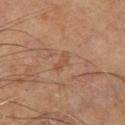The lesion is on the right leg.
A male subject, aged 58 to 62.
A 15 mm close-up tile from a total-body photography series done for melanoma screening.